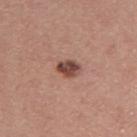Assessment:
Captured during whole-body skin photography for melanoma surveillance; the lesion was not biopsied.
Context:
A male subject approximately 40 years of age. From the right upper arm. Captured under white-light illumination. This image is a 15 mm lesion crop taken from a total-body photograph. The lesion's longest dimension is about 3 mm.